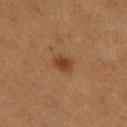Assessment:
Imaged during a routine full-body skin examination; the lesion was not biopsied and no histopathology is available.
Image and clinical context:
Cropped from a whole-body photographic skin survey; the tile spans about 15 mm. Captured under cross-polarized illumination. From the leg. Longest diameter approximately 2.5 mm. A female subject, approximately 40 years of age. An algorithmic analysis of the crop reported an area of roughly 4.5 mm², an eccentricity of roughly 0.65, and a shape-asymmetry score of about 0.25 (0 = symmetric). The analysis additionally found a lesion color around L≈33 a*≈18 b*≈29 in CIELAB and a normalized lesion–skin contrast near 7.5. The analysis additionally found a border-irregularity rating of about 2/10, internal color variation of about 2.5 on a 0–10 scale, and a peripheral color-asymmetry measure near 1.